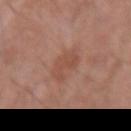{"biopsy_status": "not biopsied; imaged during a skin examination", "lesion_size": {"long_diameter_mm_approx": 3.0}, "automated_metrics": {"cielab_L": 50, "cielab_a": 23, "cielab_b": 28, "vs_skin_darker_L": 6.0, "vs_skin_contrast_norm": 5.0, "border_irregularity_0_10": 4.5, "color_variation_0_10": 1.5, "peripheral_color_asymmetry": 0.5, "nevus_likeness_0_100": 0, "lesion_detection_confidence_0_100": 100}, "lighting": "white-light", "patient": {"sex": "male", "age_approx": 70}, "image": {"source": "total-body photography crop", "field_of_view_mm": 15}, "site": "arm"}